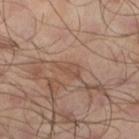This lesion was catalogued during total-body skin photography and was not selected for biopsy. Longest diameter approximately 3 mm. A region of skin cropped from a whole-body photographic capture, roughly 15 mm wide. The subject is a male roughly 65 years of age. Located on the left lower leg. Imaged with cross-polarized lighting.